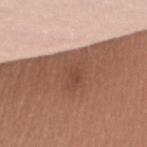| field | value |
|---|---|
| workup | imaged on a skin check; not biopsied |
| imaging modality | ~15 mm crop, total-body skin-cancer survey |
| tile lighting | white-light illumination |
| anatomic site | the left upper arm |
| subject | female, aged approximately 60 |